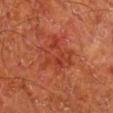automated lesion analysis: an area of roughly 13 mm², a shape eccentricity near 0.5, and a symmetry-axis asymmetry near 0.4; a classifier nevus-likeness of about 30/100 and a lesion-detection confidence of about 100/100
subject: male, aged approximately 65
body site: the leg
image source: 15 mm crop, total-body photography
size: ≈5 mm